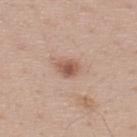The total-body-photography lesion software estimated a within-lesion color-variation index near 3.5/10 and radial color variation of about 1.
A male subject aged approximately 40.
The lesion is located on the upper back.
The tile uses white-light illumination.
About 2.5 mm across.
This image is a 15 mm lesion crop taken from a total-body photograph.